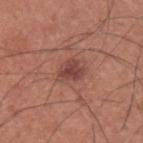notes: no biopsy performed (imaged during a skin exam) | size: about 3 mm | image-analysis metrics: a footprint of about 5.5 mm², a shape eccentricity near 0.65, and a shape-asymmetry score of about 0.35 (0 = symmetric); an automated nevus-likeness rating near 75 out of 100 and lesion-presence confidence of about 100/100 | subject: male, aged around 35 | location: the back | lighting: white-light illumination | acquisition: ~15 mm tile from a whole-body skin photo.